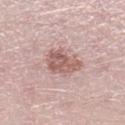notes = catalogued during a skin exam; not biopsied | body site = the left lower leg | image = ~15 mm crop, total-body skin-cancer survey | patient = male, aged 48–52.